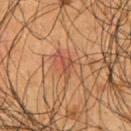biopsy status: imaged on a skin check; not biopsied
illumination: cross-polarized illumination
acquisition: ~15 mm crop, total-body skin-cancer survey
subject: male, aged 53–57
body site: the front of the torso
size: about 3.5 mm
image-analysis metrics: a lesion color around L≈46 a*≈24 b*≈31 in CIELAB, roughly 8 lightness units darker than nearby skin, and a lesion-to-skin contrast of about 6 (normalized; higher = more distinct); border irregularity of about 7 on a 0–10 scale, internal color variation of about 1 on a 0–10 scale, and radial color variation of about 0.5